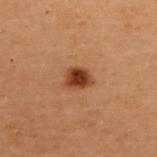Context: Captured under cross-polarized illumination. Located on the upper back. A female subject, aged 48–52. The lesion's longest dimension is about 2.5 mm. Cropped from a total-body skin-imaging series; the visible field is about 15 mm.